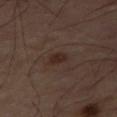Captured during whole-body skin photography for melanoma surveillance; the lesion was not biopsied. The lesion is on the right thigh. An algorithmic analysis of the crop reported a lesion area of about 3.5 mm², a shape eccentricity near 0.75, and a shape-asymmetry score of about 0.2 (0 = symmetric). The software also gave an average lesion color of about L≈26 a*≈16 b*≈20 (CIELAB) and a normalized border contrast of about 7. And it measured internal color variation of about 3 on a 0–10 scale and radial color variation of about 1. A lesion tile, about 15 mm wide, cut from a 3D total-body photograph. The lesion's longest dimension is about 2.5 mm.